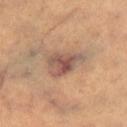workup: total-body-photography surveillance lesion; no biopsy
site: the right thigh
TBP lesion metrics: an average lesion color of about L≈50 a*≈18 b*≈24 (CIELAB), a lesion–skin lightness drop of about 11, and a normalized lesion–skin contrast near 9; a border-irregularity index near 4/10, internal color variation of about 6 on a 0–10 scale, and peripheral color asymmetry of about 2; a classifier nevus-likeness of about 0/100
acquisition: ~15 mm tile from a whole-body skin photo
tile lighting: cross-polarized illumination
subject: female, in their 30s